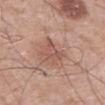Q: Was this lesion biopsied?
A: catalogued during a skin exam; not biopsied